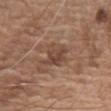Q: Was this lesion biopsied?
A: catalogued during a skin exam; not biopsied
Q: What are the patient's age and sex?
A: male, in their mid- to late 70s
Q: Lesion location?
A: the chest
Q: What is the imaging modality?
A: 15 mm crop, total-body photography